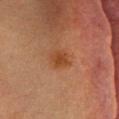Impression:
Captured during whole-body skin photography for melanoma surveillance; the lesion was not biopsied.
Image and clinical context:
This image is a 15 mm lesion crop taken from a total-body photograph. On the head or neck. Longest diameter approximately 3 mm. A female patient, aged approximately 30.